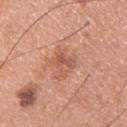| field | value |
|---|---|
| biopsy status | total-body-photography surveillance lesion; no biopsy |
| subject | male, aged approximately 30 |
| lesion diameter | about 4 mm |
| tile lighting | white-light illumination |
| TBP lesion metrics | border irregularity of about 4 on a 0–10 scale, a color-variation rating of about 5/10, and peripheral color asymmetry of about 2; a classifier nevus-likeness of about 5/100 and a detector confidence of about 100 out of 100 that the crop contains a lesion |
| site | the upper back |
| image | total-body-photography crop, ~15 mm field of view |